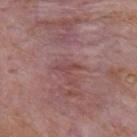The lesion was tiled from a total-body skin photograph and was not biopsied. Measured at roughly 3 mm in maximum diameter. Captured under white-light illumination. A roughly 15 mm field-of-view crop from a total-body skin photograph. The patient is a male in their mid- to late 70s. The lesion is on the mid back.